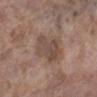<lesion>
  <biopsy_status>not biopsied; imaged during a skin examination</biopsy_status>
  <image>
    <source>total-body photography crop</source>
    <field_of_view_mm>15</field_of_view_mm>
  </image>
  <site>leg</site>
  <lesion_size>
    <long_diameter_mm_approx>5.0</long_diameter_mm_approx>
  </lesion_size>
  <automated_metrics>
    <nevus_likeness_0_100>0</nevus_likeness_0_100>
    <lesion_detection_confidence_0_100>100</lesion_detection_confidence_0_100>
  </automated_metrics>
  <lighting>white-light</lighting>
  <patient>
    <sex>female</sex>
    <age_approx>85+</age_approx>
  </patient>
</lesion>